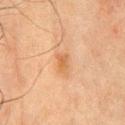{"biopsy_status": "not biopsied; imaged during a skin examination", "lighting": "cross-polarized", "site": "chest", "image": {"source": "total-body photography crop", "field_of_view_mm": 15}, "patient": {"sex": "male", "age_approx": 70}}